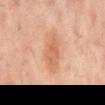Q: Was this lesion biopsied?
A: catalogued during a skin exam; not biopsied
Q: What are the patient's age and sex?
A: male, approximately 60 years of age
Q: What is the anatomic site?
A: the mid back
Q: Automated lesion metrics?
A: a footprint of about 10 mm² and an outline eccentricity of about 0.9 (0 = round, 1 = elongated); an average lesion color of about L≈59 a*≈22 b*≈34 (CIELAB), about 8 CIELAB-L* units darker than the surrounding skin, and a normalized border contrast of about 6.5; internal color variation of about 2.5 on a 0–10 scale and a peripheral color-asymmetry measure near 0.5; a nevus-likeness score of about 65/100
Q: What is the lesion's diameter?
A: ≈5 mm
Q: How was the tile lit?
A: cross-polarized illumination
Q: What is the imaging modality?
A: 15 mm crop, total-body photography